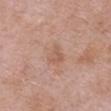Assessment: The lesion was tiled from a total-body skin photograph and was not biopsied. Background: The total-body-photography lesion software estimated an area of roughly 5 mm², a shape eccentricity near 0.6, and a shape-asymmetry score of about 0.35 (0 = symmetric). It also reported an average lesion color of about L≈58 a*≈20 b*≈29 (CIELAB), about 6 CIELAB-L* units darker than the surrounding skin, and a normalized lesion–skin contrast near 5. It also reported a border-irregularity rating of about 3/10. The software also gave a nevus-likeness score of about 0/100 and a detector confidence of about 100 out of 100 that the crop contains a lesion. The lesion is located on the chest. A 15 mm close-up extracted from a 3D total-body photography capture. A female patient, roughly 70 years of age.